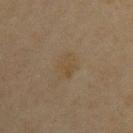notes = catalogued during a skin exam; not biopsied | image = ~15 mm crop, total-body skin-cancer survey | anatomic site = the arm | automated metrics = a footprint of about 4.5 mm² and a symmetry-axis asymmetry near 0.3; border irregularity of about 3 on a 0–10 scale, a color-variation rating of about 2/10, and peripheral color asymmetry of about 0.5; a classifier nevus-likeness of about 5/100 and lesion-presence confidence of about 100/100 | diameter = ≈3 mm | patient = female, aged approximately 45 | lighting = cross-polarized illumination.